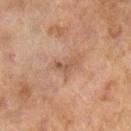Notes:
- follow-up — total-body-photography surveillance lesion; no biopsy
- anatomic site — the right lower leg
- illumination — cross-polarized illumination
- subject — female, aged 58–62
- imaging modality — ~15 mm crop, total-body skin-cancer survey